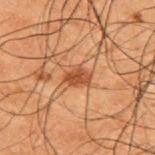<case>
  <biopsy_status>not biopsied; imaged during a skin examination</biopsy_status>
  <image>
    <source>total-body photography crop</source>
    <field_of_view_mm>15</field_of_view_mm>
  </image>
  <patient>
    <sex>male</sex>
    <age_approx>50</age_approx>
  </patient>
  <site>upper back</site>
  <automated_metrics>
    <area_mm2_approx>4.0</area_mm2_approx>
    <eccentricity>0.7</eccentricity>
    <color_variation_0_10>3.0</color_variation_0_10>
    <peripheral_color_asymmetry>1.0</peripheral_color_asymmetry>
  </automated_metrics>
  <lighting>cross-polarized</lighting>
</case>